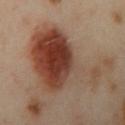* notes — no biopsy performed (imaged during a skin exam)
* image — total-body-photography crop, ~15 mm field of view
* lighting — cross-polarized
* subject — female, in their 40s
* body site — the left arm
* diameter — ≈11.5 mm